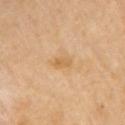The lesion was photographed on a routine skin check and not biopsied; there is no pathology result.
The lesion's longest dimension is about 2.5 mm.
This is a cross-polarized tile.
A female subject roughly 60 years of age.
A 15 mm close-up tile from a total-body photography series done for melanoma screening.
From the left arm.